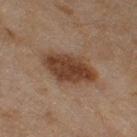Assessment:
This lesion was catalogued during total-body skin photography and was not selected for biopsy.
Clinical summary:
The subject is a female roughly 60 years of age. This is a cross-polarized tile. On the right thigh. The lesion-visualizer software estimated a mean CIELAB color near L≈36 a*≈17 b*≈27 and a normalized border contrast of about 10.5. It also reported lesion-presence confidence of about 100/100. A roughly 15 mm field-of-view crop from a total-body skin photograph. The lesion's longest dimension is about 6 mm.